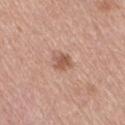<tbp_lesion>
<biopsy_status>not biopsied; imaged during a skin examination</biopsy_status>
<lighting>white-light</lighting>
<site>right thigh</site>
<lesion_size>
  <long_diameter_mm_approx>2.5</long_diameter_mm_approx>
</lesion_size>
<patient>
  <sex>female</sex>
  <age_approx>65</age_approx>
</patient>
<image>
  <source>total-body photography crop</source>
  <field_of_view_mm>15</field_of_view_mm>
</image>
<automated_metrics>
  <vs_skin_darker_L>11.0</vs_skin_darker_L>
  <vs_skin_contrast_norm>7.5</vs_skin_contrast_norm>
  <border_irregularity_0_10>2.5</border_irregularity_0_10>
  <color_variation_0_10>2.5</color_variation_0_10>
  <peripheral_color_asymmetry>0.5</peripheral_color_asymmetry>
</automated_metrics>
</tbp_lesion>